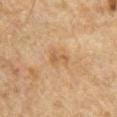| key | value |
|---|---|
| location | the chest |
| acquisition | total-body-photography crop, ~15 mm field of view |
| subject | male, about 50 years old |